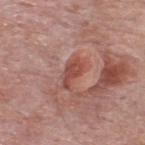{"biopsy_status": "not biopsied; imaged during a skin examination", "image": {"source": "total-body photography crop", "field_of_view_mm": 15}, "site": "upper back", "automated_metrics": {"area_mm2_approx": 4.5, "shape_asymmetry": 0.4, "border_irregularity_0_10": 4.0, "color_variation_0_10": 1.5, "peripheral_color_asymmetry": 0.5}, "patient": {"sex": "male", "age_approx": 55}, "lesion_size": {"long_diameter_mm_approx": 3.0}, "lighting": "white-light"}